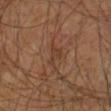  biopsy_status: not biopsied; imaged during a skin examination
  lesion_size:
    long_diameter_mm_approx: 3.0
  site: left arm
  lighting: cross-polarized
  automated_metrics:
    vs_skin_darker_L: 6.0
    vs_skin_contrast_norm: 5.5
    border_irregularity_0_10: 6.0
    peripheral_color_asymmetry: 0.5
  patient:
    sex: male
    age_approx: 65
  image:
    source: total-body photography crop
    field_of_view_mm: 15